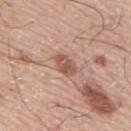The patient is a male roughly 75 years of age.
Located on the mid back.
A region of skin cropped from a whole-body photographic capture, roughly 15 mm wide.
This is a white-light tile.